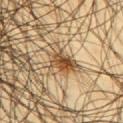biopsy status: total-body-photography surveillance lesion; no biopsy
anatomic site: the mid back
size: ≈5.5 mm
patient: male, in their mid-40s
image-analysis metrics: a lesion color around L≈52 a*≈16 b*≈35 in CIELAB, roughly 13 lightness units darker than nearby skin, and a normalized lesion–skin contrast near 9; a border-irregularity index near 5.5/10, internal color variation of about 8.5 on a 0–10 scale, and peripheral color asymmetry of about 2.5; an automated nevus-likeness rating near 85 out of 100
acquisition: ~15 mm tile from a whole-body skin photo